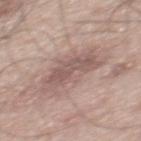notes: total-body-photography surveillance lesion; no biopsy.